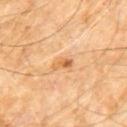follow-up: no biopsy performed (imaged during a skin exam) | lighting: cross-polarized illumination | location: the chest | subject: male, in their 60s | automated lesion analysis: about 10 CIELAB-L* units darker than the surrounding skin and a lesion-to-skin contrast of about 7 (normalized; higher = more distinct); border irregularity of about 4.5 on a 0–10 scale, internal color variation of about 1.5 on a 0–10 scale, and peripheral color asymmetry of about 0; a nevus-likeness score of about 20/100 and lesion-presence confidence of about 100/100 | image source: ~15 mm crop, total-body skin-cancer survey | lesion diameter: ≈2.5 mm.